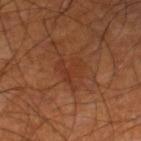| feature | finding |
|---|---|
| notes | no biopsy performed (imaged during a skin exam) |
| tile lighting | cross-polarized illumination |
| acquisition | total-body-photography crop, ~15 mm field of view |
| patient | male, approximately 60 years of age |
| location | the right lower leg |
| automated lesion analysis | an area of roughly 7 mm² and a shape-asymmetry score of about 0.6 (0 = symmetric); a lesion–skin lightness drop of about 5 and a lesion-to-skin contrast of about 5 (normalized; higher = more distinct); a border-irregularity index near 8/10, a within-lesion color-variation index near 2/10, and peripheral color asymmetry of about 0.5; a classifier nevus-likeness of about 0/100 and a detector confidence of about 95 out of 100 that the crop contains a lesion |